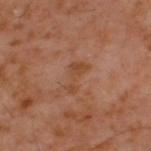biopsy_status: not biopsied; imaged during a skin examination
site: back
image:
  source: total-body photography crop
  field_of_view_mm: 15
lighting: cross-polarized
lesion_size:
  long_diameter_mm_approx: 3.5
patient:
  sex: male
  age_approx: 60